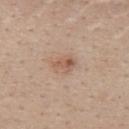Q: Is there a histopathology result?
A: imaged on a skin check; not biopsied
Q: What is the lesion's diameter?
A: ~3 mm (longest diameter)
Q: Patient demographics?
A: female, in their mid-30s
Q: What did automated image analysis measure?
A: an average lesion color of about L≈57 a*≈19 b*≈29 (CIELAB) and a lesion-to-skin contrast of about 6 (normalized; higher = more distinct); an automated nevus-likeness rating near 60 out of 100
Q: Illumination type?
A: white-light
Q: Lesion location?
A: the upper back
Q: How was this image acquired?
A: 15 mm crop, total-body photography